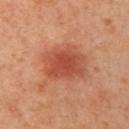image: ~15 mm crop, total-body skin-cancer survey; patient: female, about 40 years old; anatomic site: the left upper arm.